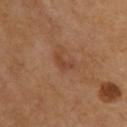{"site": "upper back", "lighting": "cross-polarized", "image": {"source": "total-body photography crop", "field_of_view_mm": 15}}